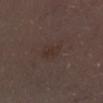Notes:
– follow-up — total-body-photography surveillance lesion; no biopsy
– imaging modality — 15 mm crop, total-body photography
– site — the left lower leg
– subject — male, about 70 years old
– automated metrics — a lesion area of about 6 mm², an outline eccentricity of about 0.75 (0 = round, 1 = elongated), and a symmetry-axis asymmetry near 0.3; a mean CIELAB color near L≈31 a*≈14 b*≈19, roughly 4 lightness units darker than nearby skin, and a normalized lesion–skin contrast near 5; an automated nevus-likeness rating near 0 out of 100 and a detector confidence of about 100 out of 100 that the crop contains a lesion
– size — ~3.5 mm (longest diameter)